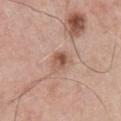{
  "biopsy_status": "not biopsied; imaged during a skin examination",
  "site": "chest",
  "image": {
    "source": "total-body photography crop",
    "field_of_view_mm": 15
  },
  "patient": {
    "sex": "male",
    "age_approx": 60
  },
  "automated_metrics": {
    "border_irregularity_0_10": 1.5,
    "color_variation_0_10": 8.0
  }
}